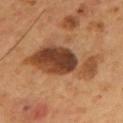Q: Was a biopsy performed?
A: no biopsy performed (imaged during a skin exam)
Q: How was the tile lit?
A: cross-polarized illumination
Q: Where on the body is the lesion?
A: the mid back
Q: What did automated image analysis measure?
A: an area of roughly 24 mm², a shape eccentricity near 0.9, and two-axis asymmetry of about 0.4; a lesion color around L≈39 a*≈21 b*≈31 in CIELAB, about 14 CIELAB-L* units darker than the surrounding skin, and a lesion-to-skin contrast of about 11 (normalized; higher = more distinct)
Q: What is the imaging modality?
A: ~15 mm tile from a whole-body skin photo
Q: What is the lesion's diameter?
A: ≈9 mm
Q: Patient demographics?
A: male, about 55 years old